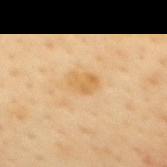Part of a total-body skin-imaging series; this lesion was reviewed on a skin check and was not flagged for biopsy.
From the mid back.
A region of skin cropped from a whole-body photographic capture, roughly 15 mm wide.
The tile uses cross-polarized illumination.
The total-body-photography lesion software estimated a footprint of about 6 mm² and an eccentricity of roughly 0.75. It also reported an average lesion color of about L≈66 a*≈17 b*≈44 (CIELAB), a lesion–skin lightness drop of about 7, and a normalized border contrast of about 6. And it measured border irregularity of about 2 on a 0–10 scale, a within-lesion color-variation index near 3/10, and radial color variation of about 1. And it measured a lesion-detection confidence of about 100/100.
The patient is a female approximately 50 years of age.
Approximately 3.5 mm at its widest.